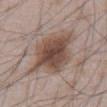This lesion was catalogued during total-body skin photography and was not selected for biopsy. The lesion is on the abdomen. This image is a 15 mm lesion crop taken from a total-body photograph. The patient is a male in their 50s.